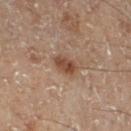workup — catalogued during a skin exam; not biopsied
image source — total-body-photography crop, ~15 mm field of view
tile lighting — cross-polarized
anatomic site — the right lower leg
size — about 3 mm
patient — male, aged around 70